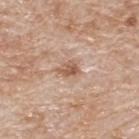The lesion was tiled from a total-body skin photograph and was not biopsied.
A 15 mm close-up tile from a total-body photography series done for melanoma screening.
A male patient aged 78 to 82.
From the back.